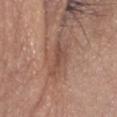Image and clinical context:
A 15 mm close-up extracted from a 3D total-body photography capture. This is a white-light tile. A male patient in their 70s. An algorithmic analysis of the crop reported a mean CIELAB color near L≈48 a*≈20 b*≈26. It also reported a border-irregularity index near 5/10, internal color variation of about 1.5 on a 0–10 scale, and radial color variation of about 0.5. It also reported a nevus-likeness score of about 0/100 and a lesion-detection confidence of about 95/100. Approximately 3.5 mm at its widest. From the head or neck.